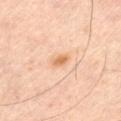Imaged during a routine full-body skin examination; the lesion was not biopsied and no histopathology is available. Approximately 2 mm at its widest. A 15 mm close-up extracted from a 3D total-body photography capture. This is a cross-polarized tile. An algorithmic analysis of the crop reported an average lesion color of about L≈66 a*≈23 b*≈38 (CIELAB), roughly 10 lightness units darker than nearby skin, and a normalized border contrast of about 7.5. The software also gave a classifier nevus-likeness of about 85/100 and a lesion-detection confidence of about 100/100. A male patient aged approximately 65. The lesion is on the left thigh.